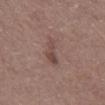Captured under white-light illumination. On the chest. The subject is a male aged 58 to 62. A roughly 15 mm field-of-view crop from a total-body skin photograph. Automated tile analysis of the lesion measured border irregularity of about 3.5 on a 0–10 scale and internal color variation of about 3.5 on a 0–10 scale. It also reported an automated nevus-likeness rating near 0 out of 100 and lesion-presence confidence of about 100/100.